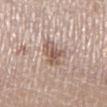Imaged during a routine full-body skin examination; the lesion was not biopsied and no histopathology is available.
The total-body-photography lesion software estimated a nevus-likeness score of about 55/100.
Cropped from a total-body skin-imaging series; the visible field is about 15 mm.
The tile uses white-light illumination.
The patient is a female aged 48–52.
On the left lower leg.